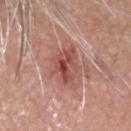Diagnosis: Histopathological examination showed a malignancy: nodular basal cell carcinoma.